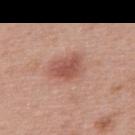| feature | finding |
|---|---|
| biopsy status | no biopsy performed (imaged during a skin exam) |
| subject | male, aged approximately 65 |
| image | total-body-photography crop, ~15 mm field of view |
| lesion diameter | ≈4 mm |
| tile lighting | white-light |
| TBP lesion metrics | a footprint of about 8.5 mm² and a symmetry-axis asymmetry near 0.25; a mean CIELAB color near L≈53 a*≈25 b*≈27, about 11 CIELAB-L* units darker than the surrounding skin, and a lesion-to-skin contrast of about 7.5 (normalized; higher = more distinct); a color-variation rating of about 3/10; a nevus-likeness score of about 95/100 and lesion-presence confidence of about 100/100 |
| anatomic site | the back |